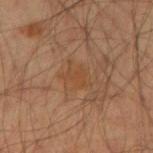| field | value |
|---|---|
| workup | no biopsy performed (imaged during a skin exam) |
| tile lighting | cross-polarized |
| site | the right upper arm |
| patient | male, about 35 years old |
| diameter | ~2.5 mm (longest diameter) |
| image | ~15 mm tile from a whole-body skin photo |